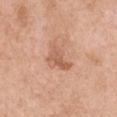The lesion is on the chest. A female subject, in their mid-50s. This is a white-light tile. A close-up tile cropped from a whole-body skin photograph, about 15 mm across. The lesion's longest dimension is about 3.5 mm. Automated image analysis of the tile measured a footprint of about 5.5 mm² and a shape eccentricity near 0.8. It also reported roughly 9 lightness units darker than nearby skin and a lesion-to-skin contrast of about 6.5 (normalized; higher = more distinct).